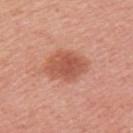The lesion was tiled from a total-body skin photograph and was not biopsied.
A 15 mm close-up extracted from a 3D total-body photography capture.
The subject is a female about 50 years old.
Imaged with white-light lighting.
About 5 mm across.
Located on the left upper arm.